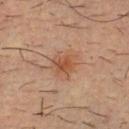biopsy status: no biopsy performed (imaged during a skin exam); patient: male, aged 33 to 37; illumination: cross-polarized; location: the chest; image: total-body-photography crop, ~15 mm field of view; lesion size: ≈3.5 mm.